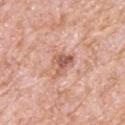Impression:
Captured during whole-body skin photography for melanoma surveillance; the lesion was not biopsied.
Clinical summary:
A region of skin cropped from a whole-body photographic capture, roughly 15 mm wide. A male patient, aged approximately 80. Approximately 3 mm at its widest. This is a white-light tile. Located on the upper back.